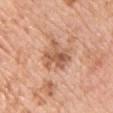Assessment: This lesion was catalogued during total-body skin photography and was not selected for biopsy. Image and clinical context: From the chest. The patient is a female about 45 years old. Imaged with white-light lighting. A 15 mm close-up extracted from a 3D total-body photography capture. The lesion-visualizer software estimated a border-irregularity rating of about 5.5/10, a color-variation rating of about 6/10, and radial color variation of about 2. The software also gave an automated nevus-likeness rating near 0 out of 100. The lesion's longest dimension is about 4 mm.